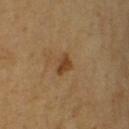{"biopsy_status": "not biopsied; imaged during a skin examination", "patient": {"sex": "male", "age_approx": 65}, "lesion_size": {"long_diameter_mm_approx": 2.5}, "site": "left upper arm", "automated_metrics": {"area_mm2_approx": 3.0, "cielab_L": 41, "cielab_a": 19, "cielab_b": 35, "vs_skin_contrast_norm": 8.5}, "lighting": "cross-polarized", "image": {"source": "total-body photography crop", "field_of_view_mm": 15}}